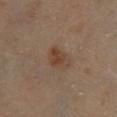The lesion was photographed on a routine skin check and not biopsied; there is no pathology result.
From the right lower leg.
This is a cross-polarized tile.
A close-up tile cropped from a whole-body skin photograph, about 15 mm across.
A male subject, aged 53–57.
Measured at roughly 3.5 mm in maximum diameter.